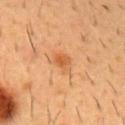Impression:
No biopsy was performed on this lesion — it was imaged during a full skin examination and was not determined to be concerning.
Clinical summary:
Captured under cross-polarized illumination. Longest diameter approximately 3 mm. A male patient, aged 53–57. On the chest. Cropped from a total-body skin-imaging series; the visible field is about 15 mm.